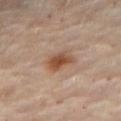<lesion>
<biopsy_status>not biopsied; imaged during a skin examination</biopsy_status>
<automated_metrics>
  <cielab_L>50</cielab_L>
  <cielab_a>19</cielab_a>
  <cielab_b>31</cielab_b>
  <border_irregularity_0_10>2.5</border_irregularity_0_10>
  <color_variation_0_10>5.0</color_variation_0_10>
  <peripheral_color_asymmetry>1.5</peripheral_color_asymmetry>
</automated_metrics>
<image>
  <source>total-body photography crop</source>
  <field_of_view_mm>15</field_of_view_mm>
</image>
<lesion_size>
  <long_diameter_mm_approx>4.0</long_diameter_mm_approx>
</lesion_size>
<patient>
  <sex>female</sex>
  <age_approx>60</age_approx>
</patient>
<lighting>cross-polarized</lighting>
<site>abdomen</site>
</lesion>